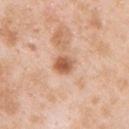Recorded during total-body skin imaging; not selected for excision or biopsy. Cropped from a whole-body photographic skin survey; the tile spans about 15 mm. From the upper back. A male patient about 25 years old. About 2.5 mm across.